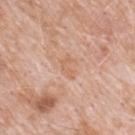• biopsy status: catalogued during a skin exam; not biopsied
• illumination: white-light illumination
• anatomic site: the upper back
• acquisition: ~15 mm tile from a whole-body skin photo
• automated metrics: a color-variation rating of about 0/10 and a peripheral color-asymmetry measure near 0
• subject: male, roughly 50 years of age